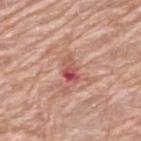Assessment:
No biopsy was performed on this lesion — it was imaged during a full skin examination and was not determined to be concerning.
Image and clinical context:
From the upper back. Imaged with white-light lighting. A 15 mm close-up extracted from a 3D total-body photography capture. Measured at roughly 3 mm in maximum diameter. The subject is a male aged 78–82.